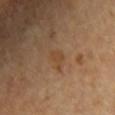Assessment: The lesion was photographed on a routine skin check and not biopsied; there is no pathology result. Acquisition and patient details: The lesion is located on the chest. Approximately 2.5 mm at its widest. A subject aged 58 to 62. The lesion-visualizer software estimated a lesion color around L≈44 a*≈17 b*≈33 in CIELAB, roughly 5 lightness units darker than nearby skin, and a normalized lesion–skin contrast near 5.5. It also reported border irregularity of about 4.5 on a 0–10 scale. Captured under cross-polarized illumination. Cropped from a whole-body photographic skin survey; the tile spans about 15 mm.